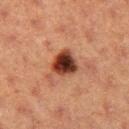{"biopsy_status": "not biopsied; imaged during a skin examination", "image": {"source": "total-body photography crop", "field_of_view_mm": 15}, "site": "left thigh", "patient": {"sex": "female", "age_approx": 40}}